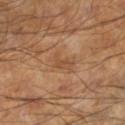{
  "biopsy_status": "not biopsied; imaged during a skin examination",
  "image": {
    "source": "total-body photography crop",
    "field_of_view_mm": 15
  },
  "site": "leg",
  "patient": {
    "sex": "male",
    "age_approx": 60
  },
  "lesion_size": {
    "long_diameter_mm_approx": 2.5
  },
  "lighting": "cross-polarized"
}